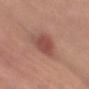follow-up: total-body-photography surveillance lesion; no biopsy.